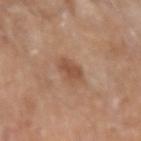Captured during whole-body skin photography for melanoma surveillance; the lesion was not biopsied. From the left upper arm. Captured under white-light illumination. Cropped from a total-body skin-imaging series; the visible field is about 15 mm. The subject is a male approximately 80 years of age.